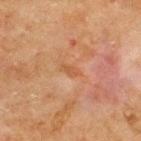The lesion was photographed on a routine skin check and not biopsied; there is no pathology result.